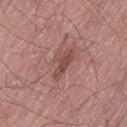Captured during whole-body skin photography for melanoma surveillance; the lesion was not biopsied. A male patient, aged approximately 65. From the lower back. Imaged with white-light lighting. An algorithmic analysis of the crop reported an average lesion color of about L≈47 a*≈22 b*≈24 (CIELAB) and a lesion-to-skin contrast of about 7 (normalized; higher = more distinct). A roughly 15 mm field-of-view crop from a total-body skin photograph. Longest diameter approximately 4 mm.